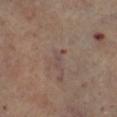biopsy_status: not biopsied; imaged during a skin examination
site: right lower leg
image:
  source: total-body photography crop
  field_of_view_mm: 15
lesion_size:
  long_diameter_mm_approx: 2.5
patient:
  sex: female
  age_approx: 60